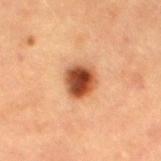notes = catalogued during a skin exam; not biopsied
illumination = cross-polarized illumination
patient = male, aged 83 or over
image = total-body-photography crop, ~15 mm field of view
site = the leg
diameter = ≈3.5 mm